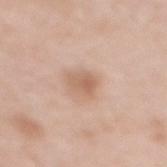* follow-up: no biopsy performed (imaged during a skin exam)
* image source: ~15 mm tile from a whole-body skin photo
* site: the mid back
* patient: female, aged 38 to 42